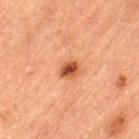The lesion was tiled from a total-body skin photograph and was not biopsied. About 2.5 mm across. A male subject, aged 83 or older. The tile uses cross-polarized illumination. This image is a 15 mm lesion crop taken from a total-body photograph. Automated image analysis of the tile measured a border-irregularity index near 2/10 and a peripheral color-asymmetry measure near 1.5. The lesion is located on the left thigh.